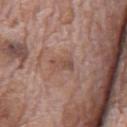This lesion was catalogued during total-body skin photography and was not selected for biopsy.
A lesion tile, about 15 mm wide, cut from a 3D total-body photograph.
A male patient roughly 70 years of age.
The lesion is located on the mid back.